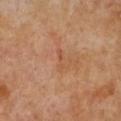Q: Was a biopsy performed?
A: catalogued during a skin exam; not biopsied
Q: What lighting was used for the tile?
A: cross-polarized illumination
Q: Who is the patient?
A: female, approximately 55 years of age
Q: How was this image acquired?
A: total-body-photography crop, ~15 mm field of view
Q: What is the anatomic site?
A: the chest
Q: What is the lesion's diameter?
A: ≈3 mm